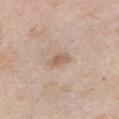biopsy status: catalogued during a skin exam; not biopsied | location: the chest | imaging modality: total-body-photography crop, ~15 mm field of view | lesion diameter: about 3 mm | subject: female, approximately 60 years of age | TBP lesion metrics: a footprint of about 3.5 mm²; a mean CIELAB color near L≈60 a*≈16 b*≈29, roughly 9 lightness units darker than nearby skin, and a lesion-to-skin contrast of about 6.5 (normalized; higher = more distinct); a border-irregularity index near 2/10 and peripheral color asymmetry of about 1; a lesion-detection confidence of about 100/100.